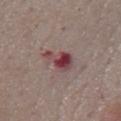Part of a total-body skin-imaging series; this lesion was reviewed on a skin check and was not flagged for biopsy.
An algorithmic analysis of the crop reported a footprint of about 6 mm², an eccentricity of roughly 0.8, and a symmetry-axis asymmetry near 0.5.
The subject is a male in their mid- to late 70s.
The lesion is located on the mid back.
The recorded lesion diameter is about 4 mm.
Captured under white-light illumination.
A region of skin cropped from a whole-body photographic capture, roughly 15 mm wide.